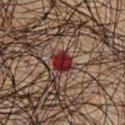The lesion was photographed on a routine skin check and not biopsied; there is no pathology result.
The subject is a male in their mid-40s.
The lesion is on the front of the torso.
This is a cross-polarized tile.
A 15 mm close-up tile from a total-body photography series done for melanoma screening.
About 2.5 mm across.
The lesion-visualizer software estimated a lesion area of about 5 mm², an eccentricity of roughly 0.5, and a symmetry-axis asymmetry near 0.15. The analysis additionally found a lesion–skin lightness drop of about 16 and a normalized border contrast of about 14.5.